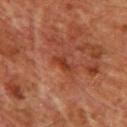Assessment: Recorded during total-body skin imaging; not selected for excision or biopsy. Image and clinical context: On the front of the torso. A 15 mm crop from a total-body photograph taken for skin-cancer surveillance. The patient is a male aged around 60. The total-body-photography lesion software estimated a lesion color around L≈35 a*≈27 b*≈31 in CIELAB, about 7 CIELAB-L* units darker than the surrounding skin, and a normalized lesion–skin contrast near 7.